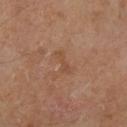Imaged during a routine full-body skin examination; the lesion was not biopsied and no histopathology is available. This is a cross-polarized tile. Located on the leg. A 15 mm close-up tile from a total-body photography series done for melanoma screening. Automated image analysis of the tile measured a normalized lesion–skin contrast near 5. And it measured a classifier nevus-likeness of about 0/100 and a detector confidence of about 100 out of 100 that the crop contains a lesion. The subject is a male roughly 65 years of age. The lesion's longest dimension is about 3 mm.